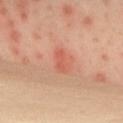| feature | finding |
|---|---|
| biopsy status | no biopsy performed (imaged during a skin exam) |
| acquisition | 15 mm crop, total-body photography |
| subject | female, in their mid-50s |
| lighting | cross-polarized illumination |
| site | the chest |
| lesion diameter | about 3 mm |